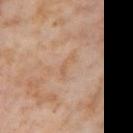Q: Is there a histopathology result?
A: no biopsy performed (imaged during a skin exam)
Q: Lesion location?
A: the right thigh
Q: How was the tile lit?
A: cross-polarized
Q: What is the lesion's diameter?
A: ~3 mm (longest diameter)
Q: Automated lesion metrics?
A: an area of roughly 2.5 mm² and an eccentricity of roughly 0.95; a border-irregularity rating of about 7/10, a within-lesion color-variation index near 0/10, and peripheral color asymmetry of about 0; a classifier nevus-likeness of about 0/100 and lesion-presence confidence of about 100/100
Q: What is the imaging modality?
A: total-body-photography crop, ~15 mm field of view
Q: What are the patient's age and sex?
A: female, in their mid- to late 50s